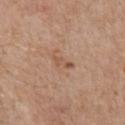The recorded lesion diameter is about 2.5 mm.
The total-body-photography lesion software estimated a border-irregularity index near 4/10, a color-variation rating of about 0/10, and peripheral color asymmetry of about 0.
The lesion is located on the chest.
This image is a 15 mm lesion crop taken from a total-body photograph.
A male patient, in their mid-60s.
This is a white-light tile.